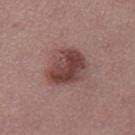follow-up: catalogued during a skin exam; not biopsied | location: the right thigh | patient: female, about 55 years old | acquisition: total-body-photography crop, ~15 mm field of view | lesion size: ≈5 mm.